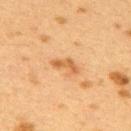Impression: Imaged during a routine full-body skin examination; the lesion was not biopsied and no histopathology is available. Context: A female patient, aged approximately 40. A region of skin cropped from a whole-body photographic capture, roughly 15 mm wide. The lesion is located on the upper back. Automated tile analysis of the lesion measured border irregularity of about 5.5 on a 0–10 scale, a color-variation rating of about 0/10, and peripheral color asymmetry of about 0.